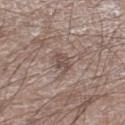{"biopsy_status": "not biopsied; imaged during a skin examination", "image": {"source": "total-body photography crop", "field_of_view_mm": 15}, "patient": {"sex": "male", "age_approx": 65}, "site": "left lower leg"}